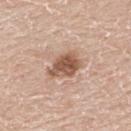notes = catalogued during a skin exam; not biopsied
size = about 4 mm
subject = male, roughly 60 years of age
acquisition = ~15 mm tile from a whole-body skin photo
lighting = white-light
site = the upper back